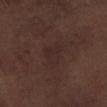Captured during whole-body skin photography for melanoma surveillance; the lesion was not biopsied. Automated image analysis of the tile measured a lesion color around L≈25 a*≈16 b*≈17 in CIELAB, a lesion–skin lightness drop of about 4, and a normalized lesion–skin contrast near 4.5. The software also gave an automated nevus-likeness rating near 5 out of 100 and a detector confidence of about 100 out of 100 that the crop contains a lesion. Cropped from a whole-body photographic skin survey; the tile spans about 15 mm. A male subject, approximately 70 years of age. The lesion is on the leg.